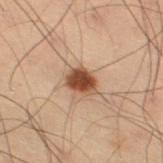Clinical impression: Captured during whole-body skin photography for melanoma surveillance; the lesion was not biopsied. Context: The lesion's longest dimension is about 3.5 mm. Cropped from a whole-body photographic skin survey; the tile spans about 15 mm. On the leg. Captured under cross-polarized illumination. The patient is a male aged around 55.